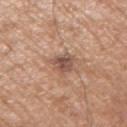The lesion was photographed on a routine skin check and not biopsied; there is no pathology result. Imaged with white-light lighting. A male subject aged around 65. On the arm. A lesion tile, about 15 mm wide, cut from a 3D total-body photograph.